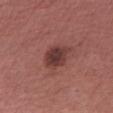Assessment: The lesion was photographed on a routine skin check and not biopsied; there is no pathology result. Clinical summary: Approximately 3.5 mm at its widest. A female patient aged 68 to 72. Cropped from a total-body skin-imaging series; the visible field is about 15 mm. From the right upper arm.